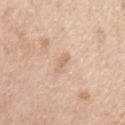Q: Was this lesion biopsied?
A: no biopsy performed (imaged during a skin exam)
Q: What are the patient's age and sex?
A: female, in their mid- to late 40s
Q: What lighting was used for the tile?
A: white-light
Q: Lesion size?
A: ~2.5 mm (longest diameter)
Q: What is the imaging modality?
A: ~15 mm tile from a whole-body skin photo
Q: What is the anatomic site?
A: the right upper arm